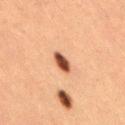subject: female, aged around 40; illumination: cross-polarized illumination; location: the right thigh; image: ~15 mm crop, total-body skin-cancer survey.